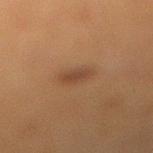This lesion was catalogued during total-body skin photography and was not selected for biopsy. Captured under cross-polarized illumination. A 15 mm close-up extracted from a 3D total-body photography capture. A female subject, approximately 40 years of age. On the leg. Automated image analysis of the tile measured a within-lesion color-variation index near 2/10 and radial color variation of about 0.5.